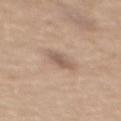Assessment:
The lesion was photographed on a routine skin check and not biopsied; there is no pathology result.
Acquisition and patient details:
The lesion is on the mid back. A close-up tile cropped from a whole-body skin photograph, about 15 mm across. The patient is a male aged 63–67.